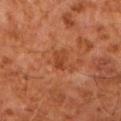Imaged during a routine full-body skin examination; the lesion was not biopsied and no histopathology is available.
The lesion is located on the right forearm.
Automated tile analysis of the lesion measured border irregularity of about 3.5 on a 0–10 scale and peripheral color asymmetry of about 0.5. It also reported a classifier nevus-likeness of about 5/100 and lesion-presence confidence of about 100/100.
The tile uses cross-polarized illumination.
A male patient, in their 60s.
A 15 mm crop from a total-body photograph taken for skin-cancer surveillance.
The lesion's longest dimension is about 3 mm.